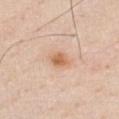| key | value |
|---|---|
| anatomic site | the front of the torso |
| imaging modality | 15 mm crop, total-body photography |
| size | ~2.5 mm (longest diameter) |
| subject | male, aged 58 to 62 |